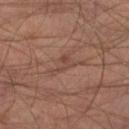Q: Who is the patient?
A: male, in their mid-30s
Q: What kind of image is this?
A: ~15 mm crop, total-body skin-cancer survey
Q: Illumination type?
A: cross-polarized
Q: What is the anatomic site?
A: the right leg
Q: What is the lesion's diameter?
A: about 3 mm
Q: Automated lesion metrics?
A: a lesion area of about 4 mm² and a shape-asymmetry score of about 0.6 (0 = symmetric); a mean CIELAB color near L≈43 a*≈19 b*≈24 and a lesion-to-skin contrast of about 5 (normalized; higher = more distinct); an automated nevus-likeness rating near 0 out of 100